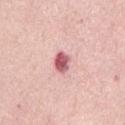Captured under white-light illumination.
A region of skin cropped from a whole-body photographic capture, roughly 15 mm wide.
A female subject approximately 55 years of age.
The lesion is on the abdomen.